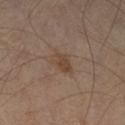workup=imaged on a skin check; not biopsied | patient=aged around 55 | image=~15 mm crop, total-body skin-cancer survey | body site=the leg.